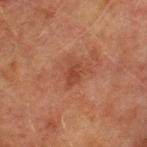Imaged during a routine full-body skin examination; the lesion was not biopsied and no histopathology is available. The lesion's longest dimension is about 2.5 mm. Located on the left upper arm. A male subject aged 73 to 77. Cropped from a total-body skin-imaging series; the visible field is about 15 mm. Captured under cross-polarized illumination.